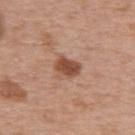Impression: The lesion was photographed on a routine skin check and not biopsied; there is no pathology result. Image and clinical context: The patient is a male about 75 years old. Captured under white-light illumination. The lesion-visualizer software estimated an average lesion color of about L≈49 a*≈23 b*≈31 (CIELAB), a lesion–skin lightness drop of about 14, and a lesion-to-skin contrast of about 9.5 (normalized; higher = more distinct). The analysis additionally found an automated nevus-likeness rating near 90 out of 100. The recorded lesion diameter is about 3 mm. A roughly 15 mm field-of-view crop from a total-body skin photograph. On the upper back.